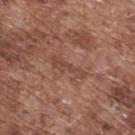Q: Was a biopsy performed?
A: no biopsy performed (imaged during a skin exam)
Q: Patient demographics?
A: male, approximately 75 years of age
Q: What kind of image is this?
A: 15 mm crop, total-body photography
Q: Lesion location?
A: the upper back
Q: What did automated image analysis measure?
A: a lesion color around L≈45 a*≈21 b*≈27 in CIELAB, a lesion–skin lightness drop of about 6, and a lesion-to-skin contrast of about 5 (normalized; higher = more distinct); a classifier nevus-likeness of about 0/100 and a detector confidence of about 75 out of 100 that the crop contains a lesion
Q: How large is the lesion?
A: ~4.5 mm (longest diameter)